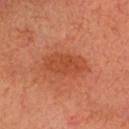Captured during whole-body skin photography for melanoma surveillance; the lesion was not biopsied. Cropped from a whole-body photographic skin survey; the tile spans about 15 mm. The patient is a male aged 58 to 62. From the head or neck.